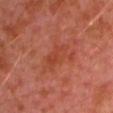Part of a total-body skin-imaging series; this lesion was reviewed on a skin check and was not flagged for biopsy. A close-up tile cropped from a whole-body skin photograph, about 15 mm across. Imaged with cross-polarized lighting. On the left upper arm. The patient is a male roughly 30 years of age.